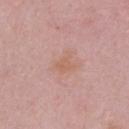Findings:
• workup · catalogued during a skin exam; not biopsied
• body site · the chest
• automated lesion analysis · an average lesion color of about L≈61 a*≈21 b*≈29 (CIELAB), roughly 5 lightness units darker than nearby skin, and a normalized border contrast of about 6; border irregularity of about 5 on a 0–10 scale, internal color variation of about 1 on a 0–10 scale, and a peripheral color-asymmetry measure near 0.5; a classifier nevus-likeness of about 0/100 and lesion-presence confidence of about 100/100
• lighting · white-light
• diameter · ~2.5 mm (longest diameter)
• subject · male, aged approximately 50
• image source · ~15 mm tile from a whole-body skin photo